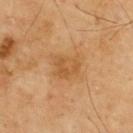biopsy_status: not biopsied; imaged during a skin examination
lesion_size:
  long_diameter_mm_approx: 4.0
patient:
  sex: male
  age_approx: 70
lighting: cross-polarized
image:
  source: total-body photography crop
  field_of_view_mm: 15
site: upper back